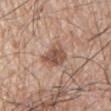The lesion was tiled from a total-body skin photograph and was not biopsied.
Imaged with white-light lighting.
Measured at roughly 3.5 mm in maximum diameter.
Cropped from a whole-body photographic skin survey; the tile spans about 15 mm.
Located on the chest.
Automated tile analysis of the lesion measured a lesion area of about 8 mm² and a shape-asymmetry score of about 0.35 (0 = symmetric). And it measured a classifier nevus-likeness of about 80/100 and lesion-presence confidence of about 100/100.
The patient is a male in their mid-60s.